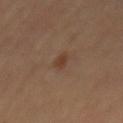Q: Is there a histopathology result?
A: no biopsy performed (imaged during a skin exam)
Q: What kind of image is this?
A: ~15 mm crop, total-body skin-cancer survey
Q: How large is the lesion?
A: ≈2.5 mm
Q: Illumination type?
A: cross-polarized
Q: Automated lesion metrics?
A: an area of roughly 2.5 mm², a shape eccentricity near 0.85, and two-axis asymmetry of about 0.45; an average lesion color of about L≈36 a*≈17 b*≈27 (CIELAB) and a normalized border contrast of about 6.5
Q: Lesion location?
A: the back
Q: Who is the patient?
A: male, aged 68–72